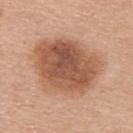Impression: Imaged during a routine full-body skin examination; the lesion was not biopsied and no histopathology is available. Clinical summary: Measured at roughly 8 mm in maximum diameter. Cropped from a whole-body photographic skin survey; the tile spans about 15 mm. Imaged with white-light lighting. Located on the upper back. The subject is a female about 40 years old.